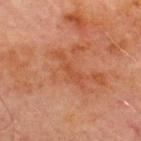| field | value |
|---|---|
| notes | no biopsy performed (imaged during a skin exam) |
| subject | male, aged around 70 |
| imaging modality | ~15 mm tile from a whole-body skin photo |
| anatomic site | the chest |